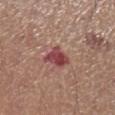Notes:
- diameter — ≈3 mm
- imaging modality — total-body-photography crop, ~15 mm field of view
- body site — the left lower leg
- illumination — white-light illumination
- patient — male, aged 63 to 67
- TBP lesion metrics — an eccentricity of roughly 0.15 and a symmetry-axis asymmetry near 0.3; a nevus-likeness score of about 5/100 and a detector confidence of about 100 out of 100 that the crop contains a lesion